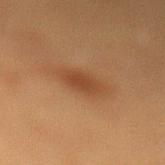patient: female, aged approximately 40
body site: the left lower leg
lesion size: about 3.5 mm
illumination: cross-polarized illumination
imaging modality: ~15 mm tile from a whole-body skin photo